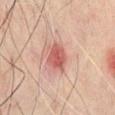Clinical impression:
The lesion was tiled from a total-body skin photograph and was not biopsied.
Background:
A male patient roughly 60 years of age. About 3.5 mm across. On the chest. Cropped from a whole-body photographic skin survey; the tile spans about 15 mm. Automated image analysis of the tile measured border irregularity of about 2 on a 0–10 scale, a color-variation rating of about 3/10, and peripheral color asymmetry of about 1. And it measured a nevus-likeness score of about 25/100 and a lesion-detection confidence of about 100/100. Captured under cross-polarized illumination.